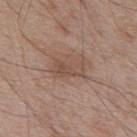Captured during whole-body skin photography for melanoma surveillance; the lesion was not biopsied. On the upper back. A 15 mm crop from a total-body photograph taken for skin-cancer surveillance. The patient is a male aged 68 to 72.